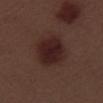notes — total-body-photography surveillance lesion; no biopsy | tile lighting — white-light | diameter — ~5 mm (longest diameter) | image — total-body-photography crop, ~15 mm field of view | TBP lesion metrics — a border-irregularity index near 1.5/10, internal color variation of about 3 on a 0–10 scale, and peripheral color asymmetry of about 1 | patient — male, aged approximately 70 | body site — the right lower leg.